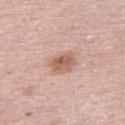workup = imaged on a skin check; not biopsied
lesion size = about 3 mm
body site = the right thigh
tile lighting = white-light illumination
subject = female, about 65 years old
image source = 15 mm crop, total-body photography